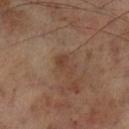Clinical impression: No biopsy was performed on this lesion — it was imaged during a full skin examination and was not determined to be concerning. Clinical summary: Imaged with cross-polarized lighting. The subject is a male aged 68 to 72. Automated image analysis of the tile measured a lesion area of about 3.5 mm², an outline eccentricity of about 0.85 (0 = round, 1 = elongated), and two-axis asymmetry of about 0.45. And it measured a lesion color around L≈39 a*≈17 b*≈27 in CIELAB, roughly 6 lightness units darker than nearby skin, and a normalized border contrast of about 5.5. The software also gave a border-irregularity rating of about 4/10 and internal color variation of about 2 on a 0–10 scale. A region of skin cropped from a whole-body photographic capture, roughly 15 mm wide. Longest diameter approximately 3 mm. From the left lower leg.